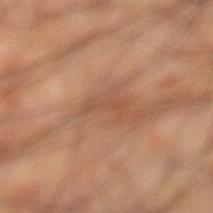Imaged during a routine full-body skin examination; the lesion was not biopsied and no histopathology is available. A roughly 15 mm field-of-view crop from a total-body skin photograph. Automated image analysis of the tile measured a lesion area of about 3 mm², a shape eccentricity near 0.95, and a shape-asymmetry score of about 0.5 (0 = symmetric). The software also gave an average lesion color of about L≈49 a*≈21 b*≈31 (CIELAB) and a lesion–skin lightness drop of about 7. The analysis additionally found lesion-presence confidence of about 65/100. On the leg. About 3.5 mm across. A male patient, aged approximately 60.